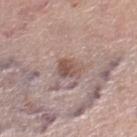Q: Was a biopsy performed?
A: total-body-photography surveillance lesion; no biopsy
Q: Automated lesion metrics?
A: an area of roughly 5 mm², a shape eccentricity near 0.4, and a shape-asymmetry score of about 0.3 (0 = symmetric); a border-irregularity rating of about 2.5/10, a color-variation rating of about 4.5/10, and radial color variation of about 1.5
Q: How large is the lesion?
A: about 2.5 mm
Q: What kind of image is this?
A: ~15 mm crop, total-body skin-cancer survey
Q: Lesion location?
A: the right lower leg
Q: What lighting was used for the tile?
A: white-light illumination
Q: Who is the patient?
A: female, aged approximately 65